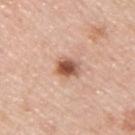biopsy status: total-body-photography surveillance lesion; no biopsy
location: the back
imaging modality: ~15 mm crop, total-body skin-cancer survey
subject: male, roughly 45 years of age